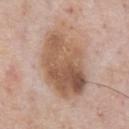Assessment:
The lesion was tiled from a total-body skin photograph and was not biopsied.
Acquisition and patient details:
This image is a 15 mm lesion crop taken from a total-body photograph. Measured at roughly 9 mm in maximum diameter. A male subject, aged 58–62. The lesion is located on the abdomen. Automated image analysis of the tile measured a color-variation rating of about 8.5/10 and peripheral color asymmetry of about 3. It also reported a classifier nevus-likeness of about 25/100 and lesion-presence confidence of about 100/100. The tile uses white-light illumination.